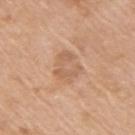The lesion was photographed on a routine skin check and not biopsied; there is no pathology result. Automated image analysis of the tile measured a footprint of about 9.5 mm², an eccentricity of roughly 0.5, and a shape-asymmetry score of about 0.2 (0 = symmetric). The software also gave a mean CIELAB color near L≈61 a*≈20 b*≈33, a lesion–skin lightness drop of about 7, and a lesion-to-skin contrast of about 5 (normalized; higher = more distinct). It also reported a border-irregularity index near 2.5/10, a color-variation rating of about 3/10, and peripheral color asymmetry of about 1. It also reported lesion-presence confidence of about 100/100. The tile uses white-light illumination. A female patient, aged approximately 75. A 15 mm close-up tile from a total-body photography series done for melanoma screening. Located on the arm.